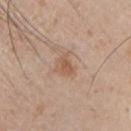tile lighting: white-light; size: ~3 mm (longest diameter); patient: male, approximately 45 years of age; image source: 15 mm crop, total-body photography; location: the chest.